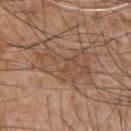The lesion was photographed on a routine skin check and not biopsied; there is no pathology result. Imaged with white-light lighting. The patient is a male aged 43–47. The lesion is on the upper back. A roughly 15 mm field-of-view crop from a total-body skin photograph. Longest diameter approximately 6.5 mm.